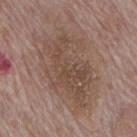Image and clinical context:
Measured at roughly 9.5 mm in maximum diameter. The lesion is on the mid back. A roughly 15 mm field-of-view crop from a total-body skin photograph. A male patient, approximately 70 years of age. Imaged with white-light lighting.